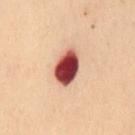{"biopsy_status": "not biopsied; imaged during a skin examination", "image": {"source": "total-body photography crop", "field_of_view_mm": 15}, "site": "mid back", "automated_metrics": {"cielab_L": 42, "cielab_a": 28, "cielab_b": 24, "vs_skin_contrast_norm": 17.0, "border_irregularity_0_10": 1.5, "color_variation_0_10": 9.0, "peripheral_color_asymmetry": 2.5, "nevus_likeness_0_100": 0}, "patient": {"sex": "female", "age_approx": 50}, "lesion_size": {"long_diameter_mm_approx": 4.5}}